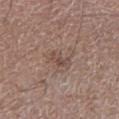follow-up=total-body-photography surveillance lesion; no biopsy | acquisition=total-body-photography crop, ~15 mm field of view | patient=male, roughly 65 years of age | lesion diameter=about 2.5 mm | image-analysis metrics=a lesion area of about 3 mm² and a shape eccentricity near 0.85; roughly 8 lightness units darker than nearby skin; border irregularity of about 5 on a 0–10 scale, internal color variation of about 0 on a 0–10 scale, and a peripheral color-asymmetry measure near 0 | location=the left lower leg | illumination=white-light.